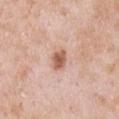• workup — total-body-photography surveillance lesion; no biopsy
• TBP lesion metrics — an eccentricity of roughly 0.8; a border-irregularity index near 2.5/10, internal color variation of about 2 on a 0–10 scale, and peripheral color asymmetry of about 0.5; a classifier nevus-likeness of about 70/100 and lesion-presence confidence of about 100/100
• lesion diameter — ≈3 mm
• acquisition — ~15 mm tile from a whole-body skin photo
• tile lighting — white-light illumination
• subject — male, aged approximately 55
• body site — the left upper arm